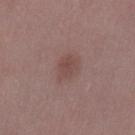  biopsy_status: not biopsied; imaged during a skin examination
  lesion_size:
    long_diameter_mm_approx: 3.5
  site: left thigh
  image:
    source: total-body photography crop
    field_of_view_mm: 15
  automated_metrics:
    cielab_L: 46
    cielab_a: 19
    cielab_b: 22
    vs_skin_darker_L: 7.0
    vs_skin_contrast_norm: 6.0
  lighting: white-light
  patient:
    sex: female
    age_approx: 45